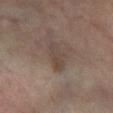biopsy status: imaged on a skin check; not biopsied | size: ≈4 mm | subject: male, roughly 70 years of age | site: the right lower leg | TBP lesion metrics: a lesion color around L≈41 a*≈12 b*≈22 in CIELAB and a lesion-to-skin contrast of about 5.5 (normalized; higher = more distinct); a border-irregularity rating of about 3.5/10, a color-variation rating of about 3.5/10, and a peripheral color-asymmetry measure near 1.5 | illumination: cross-polarized | image source: total-body-photography crop, ~15 mm field of view.